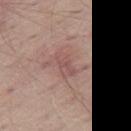{"biopsy_status": "not biopsied; imaged during a skin examination", "image": {"source": "total-body photography crop", "field_of_view_mm": 15}, "site": "right thigh", "lesion_size": {"long_diameter_mm_approx": 3.0}, "patient": {"sex": "male", "age_approx": 65}, "lighting": "white-light"}